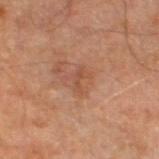The lesion was tiled from a total-body skin photograph and was not biopsied. The total-body-photography lesion software estimated a footprint of about 2 mm², an eccentricity of roughly 0.95, and a shape-asymmetry score of about 0.5 (0 = symmetric). It also reported a lesion–skin lightness drop of about 7 and a lesion-to-skin contrast of about 5 (normalized; higher = more distinct). And it measured a peripheral color-asymmetry measure near 0. The analysis additionally found a classifier nevus-likeness of about 0/100 and lesion-presence confidence of about 100/100. A male subject approximately 60 years of age. Located on the right leg. About 2.5 mm across. Cropped from a total-body skin-imaging series; the visible field is about 15 mm. Imaged with cross-polarized lighting.